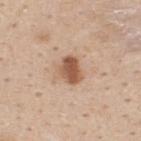Clinical impression:
No biopsy was performed on this lesion — it was imaged during a full skin examination and was not determined to be concerning.
Acquisition and patient details:
A roughly 15 mm field-of-view crop from a total-body skin photograph. On the upper back. Automated image analysis of the tile measured a lesion area of about 6.5 mm² and a symmetry-axis asymmetry near 0.25. It also reported a mean CIELAB color near L≈55 a*≈21 b*≈32, a lesion–skin lightness drop of about 15, and a normalized border contrast of about 9.5. A male patient in their 30s.